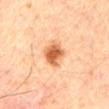No biopsy was performed on this lesion — it was imaged during a full skin examination and was not determined to be concerning. Measured at roughly 4.5 mm in maximum diameter. A male patient aged approximately 70. This is a cross-polarized tile. From the chest. This image is a 15 mm lesion crop taken from a total-body photograph.